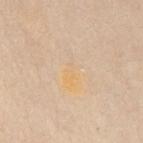subject = female, roughly 70 years of age; image = total-body-photography crop, ~15 mm field of view; location = the mid back.